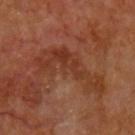The lesion was photographed on a routine skin check and not biopsied; there is no pathology result.
Captured under cross-polarized illumination.
Cropped from a whole-body photographic skin survey; the tile spans about 15 mm.
The subject is a male roughly 65 years of age.
The lesion is located on the chest.
About 8.5 mm across.